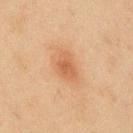Part of a total-body skin-imaging series; this lesion was reviewed on a skin check and was not flagged for biopsy.
The patient is a male in their mid-40s.
This is a cross-polarized tile.
The recorded lesion diameter is about 3.5 mm.
The lesion is located on the back.
Cropped from a total-body skin-imaging series; the visible field is about 15 mm.